The lesion is on the left forearm. The subject is a male about 55 years old. A region of skin cropped from a whole-body photographic capture, roughly 15 mm wide — 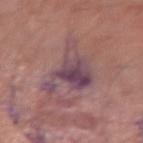Histopathologically confirmed as a squamous cell carcinoma in situ.